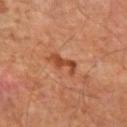Part of a total-body skin-imaging series; this lesion was reviewed on a skin check and was not flagged for biopsy. The tile uses cross-polarized illumination. The lesion-visualizer software estimated a mean CIELAB color near L≈45 a*≈27 b*≈35 and a normalized lesion–skin contrast near 8. And it measured a nevus-likeness score of about 35/100 and a detector confidence of about 100 out of 100 that the crop contains a lesion. About 3.5 mm across. A close-up tile cropped from a whole-body skin photograph, about 15 mm across. A male patient, aged 63 to 67. The lesion is located on the left upper arm.